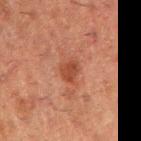The lesion was photographed on a routine skin check and not biopsied; there is no pathology result. The lesion is on the right upper arm. The recorded lesion diameter is about 2.5 mm. The tile uses cross-polarized illumination. Cropped from a total-body skin-imaging series; the visible field is about 15 mm. The subject is a male aged around 50.